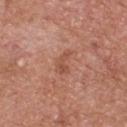notes — total-body-photography surveillance lesion; no biopsy
location — the upper back
lesion diameter — about 2.5 mm
imaging modality — total-body-photography crop, ~15 mm field of view
lighting — white-light
TBP lesion metrics — a lesion color around L≈51 a*≈25 b*≈31 in CIELAB, about 7 CIELAB-L* units darker than the surrounding skin, and a normalized border contrast of about 5.5; a border-irregularity index near 6/10, internal color variation of about 0 on a 0–10 scale, and peripheral color asymmetry of about 0
patient — male, aged approximately 75